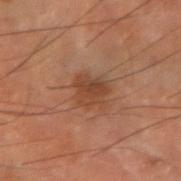Impression: The lesion was photographed on a routine skin check and not biopsied; there is no pathology result. Acquisition and patient details: A male subject about 70 years old. The recorded lesion diameter is about 3 mm. Automated image analysis of the tile measured an average lesion color of about L≈32 a*≈18 b*≈25 (CIELAB), about 7 CIELAB-L* units darker than the surrounding skin, and a normalized border contrast of about 6.5. It also reported a border-irregularity index near 3.5/10, a within-lesion color-variation index near 2.5/10, and peripheral color asymmetry of about 1. It also reported an automated nevus-likeness rating near 10 out of 100 and lesion-presence confidence of about 100/100. Captured under cross-polarized illumination. A lesion tile, about 15 mm wide, cut from a 3D total-body photograph. On the arm.